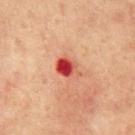Measured at roughly 3 mm in maximum diameter. Located on the abdomen. The subject is a male in their mid- to late 60s. A 15 mm close-up extracted from a 3D total-body photography capture. An algorithmic analysis of the crop reported a nevus-likeness score of about 0/100.